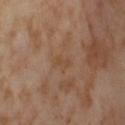notes = total-body-photography surveillance lesion; no biopsy
patient = female, aged 53 to 57
image source = ~15 mm tile from a whole-body skin photo
lighting = cross-polarized
location = the right thigh